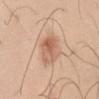Q: Is there a histopathology result?
A: imaged on a skin check; not biopsied
Q: What kind of image is this?
A: 15 mm crop, total-body photography
Q: Lesion location?
A: the right upper arm
Q: Patient demographics?
A: male, roughly 45 years of age